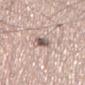Image and clinical context: A male subject aged approximately 45. Approximately 2.5 mm at its widest. On the left thigh. A roughly 15 mm field-of-view crop from a total-body skin photograph. Captured under white-light illumination.